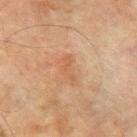This lesion was catalogued during total-body skin photography and was not selected for biopsy.